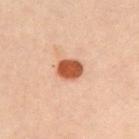The total-body-photography lesion software estimated roughly 16 lightness units darker than nearby skin and a normalized lesion–skin contrast near 12. The analysis additionally found a lesion-detection confidence of about 100/100. This is a cross-polarized tile. Longest diameter approximately 3 mm. This image is a 15 mm lesion crop taken from a total-body photograph. A female subject in their 30s. Located on the left upper arm.